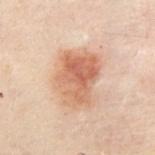| feature | finding |
|---|---|
| patient | male, aged approximately 50 |
| image source | ~15 mm crop, total-body skin-cancer survey |
| size | ~6 mm (longest diameter) |
| TBP lesion metrics | a lesion area of about 20 mm² and an outline eccentricity of about 0.7 (0 = round, 1 = elongated); an average lesion color of about L≈51 a*≈19 b*≈28 (CIELAB), a lesion–skin lightness drop of about 10, and a normalized border contrast of about 7.5; a border-irregularity index near 2.5/10, internal color variation of about 5.5 on a 0–10 scale, and radial color variation of about 2 |
| tile lighting | cross-polarized |
| location | the chest |